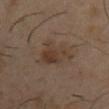Recorded during total-body skin imaging; not selected for excision or biopsy.
This is a cross-polarized tile.
An algorithmic analysis of the crop reported an area of roughly 10 mm², an outline eccentricity of about 0.8 (0 = round, 1 = elongated), and a symmetry-axis asymmetry near 0.4.
A lesion tile, about 15 mm wide, cut from a 3D total-body photograph.
The patient is a male aged 38 to 42.
The lesion's longest dimension is about 5 mm.
Located on the chest.